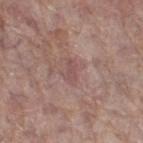Assessment: This lesion was catalogued during total-body skin photography and was not selected for biopsy. Clinical summary: A female patient aged approximately 85 or older. The tile uses white-light illumination. A region of skin cropped from a whole-body photographic capture, roughly 15 mm wide. The total-body-photography lesion software estimated internal color variation of about 1.5 on a 0–10 scale and peripheral color asymmetry of about 0.5. The analysis additionally found an automated nevus-likeness rating near 0 out of 100. The lesion's longest dimension is about 2.5 mm. Located on the right thigh.